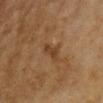A female subject, in their mid-50s.
The recorded lesion diameter is about 3.5 mm.
This is a cross-polarized tile.
Cropped from a total-body skin-imaging series; the visible field is about 15 mm.
From the right upper arm.